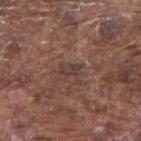biopsy status: total-body-photography surveillance lesion; no biopsy
image: ~15 mm tile from a whole-body skin photo
anatomic site: the right upper arm
patient: male, in their mid- to late 70s